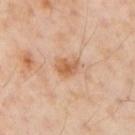Q: Is there a histopathology result?
A: catalogued during a skin exam; not biopsied
Q: Lesion location?
A: the right upper arm
Q: Illumination type?
A: cross-polarized illumination
Q: What is the imaging modality?
A: ~15 mm tile from a whole-body skin photo
Q: Who is the patient?
A: male, in their mid-50s
Q: What is the lesion's diameter?
A: about 2.5 mm
Q: Automated lesion metrics?
A: an average lesion color of about L≈60 a*≈22 b*≈37 (CIELAB) and a lesion–skin lightness drop of about 10